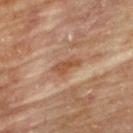Assessment: Captured during whole-body skin photography for melanoma surveillance; the lesion was not biopsied. Acquisition and patient details: The subject is a male aged 83 or older. This image is a 15 mm lesion crop taken from a total-body photograph. From the back.